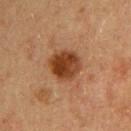follow-up=imaged on a skin check; not biopsied
acquisition=total-body-photography crop, ~15 mm field of view
diameter=about 4 mm
subject=male, aged 58 to 62
tile lighting=cross-polarized illumination
automated lesion analysis=a footprint of about 12 mm² and a shape eccentricity near 0.3; a border-irregularity rating of about 1.5/10, a color-variation rating of about 5.5/10, and radial color variation of about 2